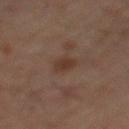The lesion was tiled from a total-body skin photograph and was not biopsied. The subject is a male aged approximately 60. A 15 mm close-up tile from a total-body photography series done for melanoma screening. An algorithmic analysis of the crop reported an average lesion color of about L≈32 a*≈16 b*≈23 (CIELAB) and a normalized lesion–skin contrast near 7. And it measured a classifier nevus-likeness of about 60/100 and a detector confidence of about 100 out of 100 that the crop contains a lesion. Captured under cross-polarized illumination. The lesion is on the mid back.